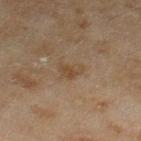Clinical impression: The lesion was tiled from a total-body skin photograph and was not biopsied. Context: The lesion-visualizer software estimated an average lesion color of about L≈41 a*≈14 b*≈29 (CIELAB) and roughly 6 lightness units darker than nearby skin. The analysis additionally found a classifier nevus-likeness of about 5/100. Captured under cross-polarized illumination. A female subject, aged 58 to 62. A 15 mm close-up extracted from a 3D total-body photography capture. Longest diameter approximately 3 mm. The lesion is on the right thigh.